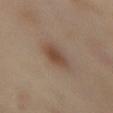follow-up: total-body-photography surveillance lesion; no biopsy
subject: female, aged approximately 55
imaging modality: total-body-photography crop, ~15 mm field of view
anatomic site: the back
size: ~3 mm (longest diameter)
illumination: cross-polarized illumination